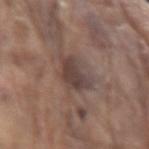Assessment:
No biopsy was performed on this lesion — it was imaged during a full skin examination and was not determined to be concerning.
Context:
From the left forearm. Automated image analysis of the tile measured a border-irregularity rating of about 3.5/10, internal color variation of about 2.5 on a 0–10 scale, and a peripheral color-asymmetry measure near 1. The lesion's longest dimension is about 4 mm. A 15 mm crop from a total-body photograph taken for skin-cancer surveillance. A male patient aged approximately 80.